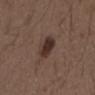This lesion was catalogued during total-body skin photography and was not selected for biopsy.
Measured at roughly 3.5 mm in maximum diameter.
This is a white-light tile.
A close-up tile cropped from a whole-body skin photograph, about 15 mm across.
A male patient roughly 50 years of age.
The lesion is located on the chest.